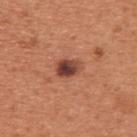The lesion was tiled from a total-body skin photograph and was not biopsied. A male subject in their mid-50s. A 15 mm crop from a total-body photograph taken for skin-cancer surveillance. The lesion is on the upper back.